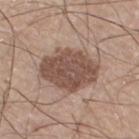Case summary:
* follow-up · no biopsy performed (imaged during a skin exam)
* subject · male, aged around 75
* acquisition · total-body-photography crop, ~15 mm field of view
* body site · the leg